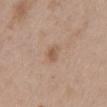The lesion was photographed on a routine skin check and not biopsied; there is no pathology result.
This is a white-light tile.
The lesion's longest dimension is about 2.5 mm.
The lesion-visualizer software estimated a lesion area of about 3 mm², a shape eccentricity near 0.85, and a symmetry-axis asymmetry near 0.3. It also reported a mean CIELAB color near L≈56 a*≈17 b*≈30, roughly 8 lightness units darker than nearby skin, and a normalized border contrast of about 6. It also reported a classifier nevus-likeness of about 5/100 and lesion-presence confidence of about 100/100.
A 15 mm close-up extracted from a 3D total-body photography capture.
A female patient, about 40 years old.
On the mid back.